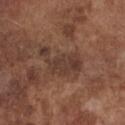patient:
  sex: male
  age_approx: 75
image:
  source: total-body photography crop
  field_of_view_mm: 15
site: chest
lighting: white-light